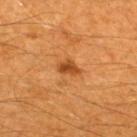No biopsy was performed on this lesion — it was imaged during a full skin examination and was not determined to be concerning.
A roughly 15 mm field-of-view crop from a total-body skin photograph.
The patient is a male roughly 60 years of age.
On the back.
Automated tile analysis of the lesion measured a footprint of about 3.5 mm², an eccentricity of roughly 0.75, and two-axis asymmetry of about 0.3. And it measured a classifier nevus-likeness of about 95/100 and a lesion-detection confidence of about 100/100.
Longest diameter approximately 2.5 mm.
This is a cross-polarized tile.